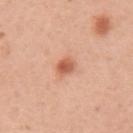Assessment:
Captured during whole-body skin photography for melanoma surveillance; the lesion was not biopsied.
Acquisition and patient details:
The lesion is on the right upper arm. Approximately 2.5 mm at its widest. Captured under white-light illumination. A close-up tile cropped from a whole-body skin photograph, about 15 mm across. A female subject, approximately 45 years of age.